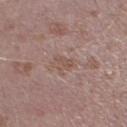Captured during whole-body skin photography for melanoma surveillance; the lesion was not biopsied. Automated tile analysis of the lesion measured a lesion area of about 4 mm² and an outline eccentricity of about 0.65 (0 = round, 1 = elongated). And it measured a border-irregularity rating of about 3.5/10, a color-variation rating of about 2.5/10, and a peripheral color-asymmetry measure near 1. It also reported a nevus-likeness score of about 0/100 and a lesion-detection confidence of about 100/100. On the left lower leg. A male patient aged approximately 40. Captured under white-light illumination. Cropped from a total-body skin-imaging series; the visible field is about 15 mm.